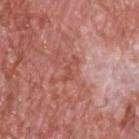{"automated_metrics": {"area_mm2_approx": 3.5, "cielab_L": 51, "cielab_a": 28, "cielab_b": 28, "vs_skin_darker_L": 7.0, "border_irregularity_0_10": 5.5, "peripheral_color_asymmetry": 0.0, "nevus_likeness_0_100": 0, "lesion_detection_confidence_0_100": 95}, "patient": {"sex": "male", "age_approx": 60}, "lighting": "white-light", "image": {"source": "total-body photography crop", "field_of_view_mm": 15}, "lesion_size": {"long_diameter_mm_approx": 3.5}, "site": "upper back"}